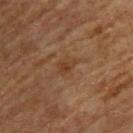This lesion was catalogued during total-body skin photography and was not selected for biopsy.
A 15 mm crop from a total-body photograph taken for skin-cancer surveillance.
The lesion is on the upper back.
About 2.5 mm across.
A male patient aged around 65.
This is a cross-polarized tile.